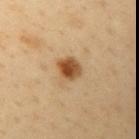Assessment: Recorded during total-body skin imaging; not selected for excision or biopsy. Context: The lesion's longest dimension is about 3 mm. A male subject aged 38 to 42. The lesion is on the left upper arm. Automated tile analysis of the lesion measured a footprint of about 6 mm², a shape eccentricity near 0.45, and a symmetry-axis asymmetry near 0.15. It also reported a mean CIELAB color near L≈51 a*≈21 b*≈39, a lesion–skin lightness drop of about 15, and a lesion-to-skin contrast of about 11 (normalized; higher = more distinct). It also reported a border-irregularity rating of about 1.5/10, a color-variation rating of about 7/10, and radial color variation of about 2.5. Cropped from a total-body skin-imaging series; the visible field is about 15 mm.